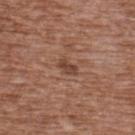{
  "biopsy_status": "not biopsied; imaged during a skin examination",
  "lighting": "white-light",
  "automated_metrics": {
    "area_mm2_approx": 3.0,
    "shape_asymmetry": 0.35,
    "cielab_L": 43,
    "cielab_a": 21,
    "cielab_b": 27,
    "vs_skin_darker_L": 9.0,
    "border_irregularity_0_10": 3.0,
    "peripheral_color_asymmetry": 0.5,
    "nevus_likeness_0_100": 0,
    "lesion_detection_confidence_0_100": 100
  },
  "image": {
    "source": "total-body photography crop",
    "field_of_view_mm": 15
  },
  "patient": {
    "sex": "male",
    "age_approx": 75
  },
  "site": "upper back",
  "lesion_size": {
    "long_diameter_mm_approx": 2.5
  }
}